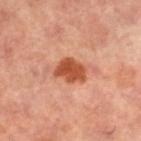site = the leg; subject = female, aged around 60; image = ~15 mm crop, total-body skin-cancer survey.